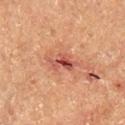Notes:
– follow-up — no biopsy performed (imaged during a skin exam)
– body site — the right lower leg
– subject — male, aged approximately 65
– image source — ~15 mm tile from a whole-body skin photo
– lighting — cross-polarized
– lesion diameter — ≈4 mm